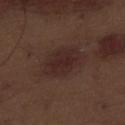workup = imaged on a skin check; not biopsied
illumination = white-light illumination
size = ≈6 mm
site = the abdomen
patient = male, in their 70s
TBP lesion metrics = a lesion color around L≈26 a*≈17 b*≈19 in CIELAB and a normalized lesion–skin contrast near 7.5; border irregularity of about 2 on a 0–10 scale, a within-lesion color-variation index near 3/10, and a peripheral color-asymmetry measure near 1; a lesion-detection confidence of about 100/100
imaging modality = ~15 mm crop, total-body skin-cancer survey